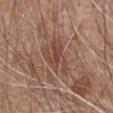<case>
  <biopsy_status>not biopsied; imaged during a skin examination</biopsy_status>
  <site>back</site>
  <patient>
    <sex>male</sex>
    <age_approx>60</age_approx>
  </patient>
  <image>
    <source>total-body photography crop</source>
    <field_of_view_mm>15</field_of_view_mm>
  </image>
</case>